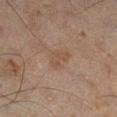No biopsy was performed on this lesion — it was imaged during a full skin examination and was not determined to be concerning. Measured at roughly 3 mm in maximum diameter. A 15 mm crop from a total-body photograph taken for skin-cancer surveillance. The lesion is on the right lower leg. A male patient, aged approximately 45.